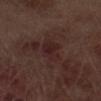The lesion was tiled from a total-body skin photograph and was not biopsied. About 4 mm across. From the leg. Captured under white-light illumination. A male patient about 70 years old. A lesion tile, about 15 mm wide, cut from a 3D total-body photograph.